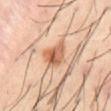| key | value |
|---|---|
| biopsy status | imaged on a skin check; not biopsied |
| imaging modality | ~15 mm crop, total-body skin-cancer survey |
| site | the abdomen |
| patient | male, approximately 40 years of age |
| illumination | cross-polarized |